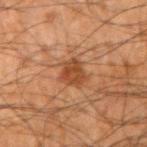workup: catalogued during a skin exam; not biopsied
body site: the right upper arm
subject: male, aged 63 to 67
imaging modality: ~15 mm crop, total-body skin-cancer survey
lesion diameter: ~3.5 mm (longest diameter)